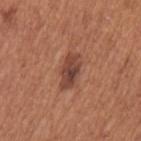Assessment:
Captured during whole-body skin photography for melanoma surveillance; the lesion was not biopsied.
Image and clinical context:
On the left upper arm. A female subject about 65 years old. A close-up tile cropped from a whole-body skin photograph, about 15 mm across.